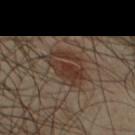illumination=cross-polarized | automated metrics=a border-irregularity rating of about 3/10 and internal color variation of about 4.5 on a 0–10 scale; a classifier nevus-likeness of about 75/100 and a detector confidence of about 100 out of 100 that the crop contains a lesion | image=~15 mm tile from a whole-body skin photo | anatomic site=the front of the torso | lesion size=about 5 mm | patient=male, aged around 65.